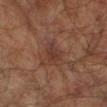Imaged with cross-polarized lighting.
The lesion's longest dimension is about 2.5 mm.
A male patient aged approximately 65.
The lesion is on the left forearm.
A 15 mm close-up extracted from a 3D total-body photography capture.